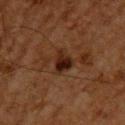Cropped from a total-body skin-imaging series; the visible field is about 15 mm. The tile uses cross-polarized illumination. The lesion is located on the upper back. Approximately 3 mm at its widest. A male patient, in their mid- to late 60s.